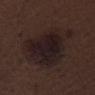The lesion was photographed on a routine skin check and not biopsied; there is no pathology result. The patient is a male aged approximately 70. This image is a 15 mm lesion crop taken from a total-body photograph. Located on the leg.